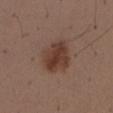<record>
  <biopsy_status>not biopsied; imaged during a skin examination</biopsy_status>
  <image>
    <source>total-body photography crop</source>
    <field_of_view_mm>15</field_of_view_mm>
  </image>
  <site>abdomen</site>
  <patient>
    <sex>male</sex>
    <age_approx>40</age_approx>
  </patient>
</record>